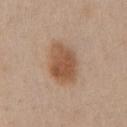  biopsy_status: not biopsied; imaged during a skin examination
  image:
    source: total-body photography crop
    field_of_view_mm: 15
  lighting: white-light
  lesion_size:
    long_diameter_mm_approx: 5.0
  patient:
    sex: male
    age_approx: 60
  site: chest